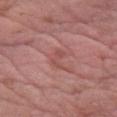follow-up: total-body-photography surveillance lesion; no biopsy | body site: the left forearm | patient: male, in their mid-50s | imaging modality: total-body-photography crop, ~15 mm field of view | automated metrics: a lesion area of about 3 mm² and a shape-asymmetry score of about 0.6 (0 = symmetric); an average lesion color of about L≈51 a*≈26 b*≈24 (CIELAB), about 7 CIELAB-L* units darker than the surrounding skin, and a lesion-to-skin contrast of about 5.5 (normalized; higher = more distinct); border irregularity of about 7 on a 0–10 scale, a color-variation rating of about 0/10, and a peripheral color-asymmetry measure near 0 | lesion size: about 3 mm.